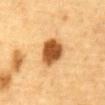No biopsy was performed on this lesion — it was imaged during a full skin examination and was not determined to be concerning.